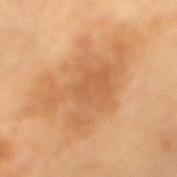Assessment: Imaged during a routine full-body skin examination; the lesion was not biopsied and no histopathology is available. Acquisition and patient details: A female patient, aged 68 to 72. A region of skin cropped from a whole-body photographic capture, roughly 15 mm wide. From the mid back.